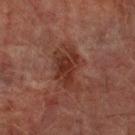Impression:
The lesion was photographed on a routine skin check and not biopsied; there is no pathology result.
Image and clinical context:
This image is a 15 mm lesion crop taken from a total-body photograph. The patient is a male approximately 75 years of age. Automated image analysis of the tile measured an area of roughly 14 mm², an outline eccentricity of about 0.75 (0 = round, 1 = elongated), and a symmetry-axis asymmetry near 0.35. The software also gave a lesion color around L≈27 a*≈20 b*≈22 in CIELAB, about 7 CIELAB-L* units darker than the surrounding skin, and a lesion-to-skin contrast of about 7.5 (normalized; higher = more distinct). Longest diameter approximately 5.5 mm. The lesion is located on the arm.